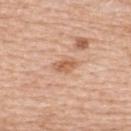An algorithmic analysis of the crop reported a mean CIELAB color near L≈60 a*≈23 b*≈35 and about 10 CIELAB-L* units darker than the surrounding skin. It also reported border irregularity of about 3 on a 0–10 scale, a within-lesion color-variation index near 3/10, and a peripheral color-asymmetry measure near 1. A 15 mm close-up tile from a total-body photography series done for melanoma screening. From the upper back. This is a white-light tile. The patient is a female approximately 60 years of age. The lesion's longest dimension is about 3 mm.